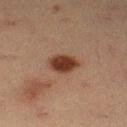Recorded during total-body skin imaging; not selected for excision or biopsy.
A 15 mm close-up tile from a total-body photography series done for melanoma screening.
The lesion-visualizer software estimated an automated nevus-likeness rating near 100 out of 100 and a lesion-detection confidence of about 100/100.
The tile uses cross-polarized illumination.
A female patient in their mid- to late 50s.
Longest diameter approximately 3.5 mm.
From the leg.